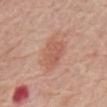This lesion was catalogued during total-body skin photography and was not selected for biopsy. Imaged with white-light lighting. A 15 mm close-up extracted from a 3D total-body photography capture. About 4.5 mm across. Located on the abdomen. An algorithmic analysis of the crop reported a nevus-likeness score of about 90/100 and lesion-presence confidence of about 100/100. A male subject approximately 80 years of age.